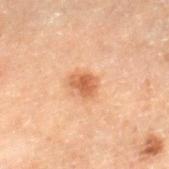workup: total-body-photography surveillance lesion; no biopsy | subject: male, approximately 70 years of age | image-analysis metrics: a footprint of about 4.5 mm² and an outline eccentricity of about 0.65 (0 = round, 1 = elongated); a lesion color around L≈46 a*≈21 b*≈31 in CIELAB and a lesion–skin lightness drop of about 10; a border-irregularity rating of about 2/10 and a within-lesion color-variation index near 2/10 | site: the right lower leg | illumination: cross-polarized illumination | lesion diameter: ≈2.5 mm | imaging modality: ~15 mm crop, total-body skin-cancer survey.